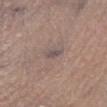Impression: No biopsy was performed on this lesion — it was imaged during a full skin examination and was not determined to be concerning. Clinical summary: Located on the leg. A male patient aged 63–67. The tile uses white-light illumination. Automated image analysis of the tile measured a lesion area of about 2.5 mm², an eccentricity of roughly 0.9, and a shape-asymmetry score of about 0.25 (0 = symmetric). The analysis additionally found a border-irregularity rating of about 2.5/10. A roughly 15 mm field-of-view crop from a total-body skin photograph.